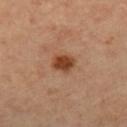Findings:
• notes — imaged on a skin check; not biopsied
• image — ~15 mm tile from a whole-body skin photo
• tile lighting — cross-polarized illumination
• automated lesion analysis — border irregularity of about 2 on a 0–10 scale, a color-variation rating of about 3/10, and peripheral color asymmetry of about 1; an automated nevus-likeness rating near 100 out of 100 and a detector confidence of about 100 out of 100 that the crop contains a lesion
• lesion diameter — about 2.5 mm
• body site — the right thigh
• patient — male, aged approximately 65